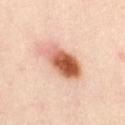The lesion is on the abdomen. A female subject, roughly 40 years of age. About 5.5 mm across. Captured under cross-polarized illumination. Cropped from a total-body skin-imaging series; the visible field is about 15 mm.